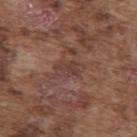A male patient in their mid- to late 70s.
The lesion is on the upper back.
Cropped from a total-body skin-imaging series; the visible field is about 15 mm.
The tile uses white-light illumination.